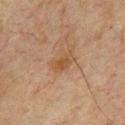No biopsy was performed on this lesion — it was imaged during a full skin examination and was not determined to be concerning.
A male subject in their mid- to late 60s.
A roughly 15 mm field-of-view crop from a total-body skin photograph.
Captured under cross-polarized illumination.
Located on the chest.
The recorded lesion diameter is about 3 mm.